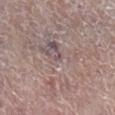Impression: Imaged during a routine full-body skin examination; the lesion was not biopsied and no histopathology is available. Context: Approximately 1.5 mm at its widest. A female patient, in their mid-60s. On the right lower leg. This image is a 15 mm lesion crop taken from a total-body photograph.